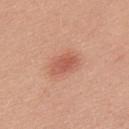Q: Was a biopsy performed?
A: imaged on a skin check; not biopsied
Q: Where on the body is the lesion?
A: the upper back
Q: Patient demographics?
A: male, aged around 25
Q: What kind of image is this?
A: ~15 mm tile from a whole-body skin photo